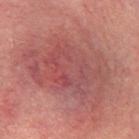Context: The tile uses cross-polarized illumination. A female subject, aged around 80. The lesion is located on the chest. Cropped from a whole-body photographic skin survey; the tile spans about 15 mm. Measured at roughly 10 mm in maximum diameter. The total-body-photography lesion software estimated border irregularity of about 3.5 on a 0–10 scale and a color-variation rating of about 6/10.